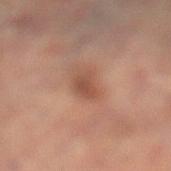Case summary:
- biopsy status: catalogued during a skin exam; not biopsied
- location: the leg
- tile lighting: cross-polarized
- image: ~15 mm crop, total-body skin-cancer survey
- subject: female, aged 78–82
- automated lesion analysis: a footprint of about 4.5 mm² and an eccentricity of roughly 0.85; a border-irregularity rating of about 3/10 and radial color variation of about 1; an automated nevus-likeness rating near 5 out of 100 and a detector confidence of about 100 out of 100 that the crop contains a lesion